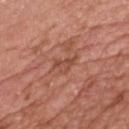Clinical impression:
Recorded during total-body skin imaging; not selected for excision or biopsy.
Clinical summary:
Automated tile analysis of the lesion measured a footprint of about 4 mm², a shape eccentricity near 0.8, and a symmetry-axis asymmetry near 0.5. It also reported border irregularity of about 5 on a 0–10 scale, internal color variation of about 2 on a 0–10 scale, and radial color variation of about 0.5. This is a white-light tile. Located on the chest. A male subject, in their 50s. A 15 mm close-up extracted from a 3D total-body photography capture.